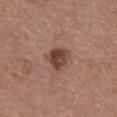Imaged during a routine full-body skin examination; the lesion was not biopsied and no histopathology is available.
Longest diameter approximately 3 mm.
This is a white-light tile.
From the chest.
A lesion tile, about 15 mm wide, cut from a 3D total-body photograph.
The subject is a female aged approximately 50.